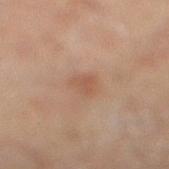This lesion was catalogued during total-body skin photography and was not selected for biopsy.
A 15 mm crop from a total-body photograph taken for skin-cancer surveillance.
The lesion-visualizer software estimated a border-irregularity index near 2.5/10, a within-lesion color-variation index near 2/10, and a peripheral color-asymmetry measure near 0.5. The software also gave a nevus-likeness score of about 15/100 and lesion-presence confidence of about 100/100.
A male subject, in their mid- to late 60s.
Located on the right lower leg.